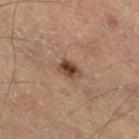{"biopsy_status": "not biopsied; imaged during a skin examination", "site": "right lower leg", "patient": {"sex": "male", "age_approx": 70}, "image": {"source": "total-body photography crop", "field_of_view_mm": 15}, "lighting": "cross-polarized", "lesion_size": {"long_diameter_mm_approx": 2.5}}